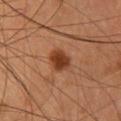Recorded during total-body skin imaging; not selected for excision or biopsy.
A region of skin cropped from a whole-body photographic capture, roughly 15 mm wide.
A female patient aged 33 to 37.
On the head or neck.